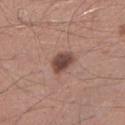Notes:
- biopsy status — no biopsy performed (imaged during a skin exam)
- image-analysis metrics — an outline eccentricity of about 0.7 (0 = round, 1 = elongated); a lesion color around L≈46 a*≈20 b*≈23 in CIELAB, about 14 CIELAB-L* units darker than the surrounding skin, and a normalized lesion–skin contrast near 10; a border-irregularity rating of about 2/10, internal color variation of about 3.5 on a 0–10 scale, and radial color variation of about 1; a classifier nevus-likeness of about 70/100 and a detector confidence of about 100 out of 100 that the crop contains a lesion
- image source — ~15 mm crop, total-body skin-cancer survey
- patient — male, roughly 55 years of age
- illumination — white-light
- lesion diameter — ≈3.5 mm
- location — the left lower leg